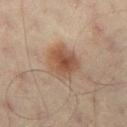Part of a total-body skin-imaging series; this lesion was reviewed on a skin check and was not flagged for biopsy. A roughly 15 mm field-of-view crop from a total-body skin photograph. A male patient, about 60 years old. The lesion is on the right thigh.